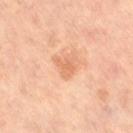tile lighting = cross-polarized illumination | patient = female, aged around 65 | image = ~15 mm crop, total-body skin-cancer survey | location = the left leg.